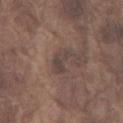Acquisition and patient details:
A male subject, in their mid- to late 70s. The lesion is on the front of the torso. A 15 mm close-up tile from a total-body photography series done for melanoma screening.